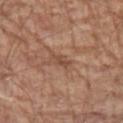Q: Was a biopsy performed?
A: total-body-photography surveillance lesion; no biopsy
Q: What are the patient's age and sex?
A: male, about 80 years old
Q: Lesion location?
A: the arm
Q: What is the lesion's diameter?
A: ≈3 mm
Q: Automated lesion metrics?
A: an average lesion color of about L≈48 a*≈20 b*≈30 (CIELAB), about 8 CIELAB-L* units darker than the surrounding skin, and a normalized border contrast of about 6; a border-irregularity index near 3.5/10, a color-variation rating of about 1.5/10, and a peripheral color-asymmetry measure near 0.5
Q: How was this image acquired?
A: 15 mm crop, total-body photography
Q: Illumination type?
A: white-light illumination